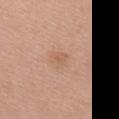{"biopsy_status": "not biopsied; imaged during a skin examination", "patient": {"sex": "female", "age_approx": 65}, "site": "chest", "image": {"source": "total-body photography crop", "field_of_view_mm": 15}, "lighting": "white-light"}